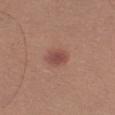Background: A male patient roughly 25 years of age. A close-up tile cropped from a whole-body skin photograph, about 15 mm across. The lesion is located on the left upper arm.